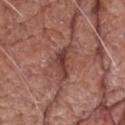Part of a total-body skin-imaging series; this lesion was reviewed on a skin check and was not flagged for biopsy.